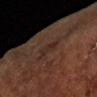  lighting: cross-polarized
  site: left arm
  lesion_size:
    long_diameter_mm_approx: 3.0
  patient:
    sex: male
    age_approx: 65
  automated_metrics:
    color_variation_0_10: 2.0
    peripheral_color_asymmetry: 0.5
    lesion_detection_confidence_0_100: 100
  image:
    source: total-body photography crop
    field_of_view_mm: 15